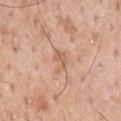notes: total-body-photography surveillance lesion; no biopsy
site: the chest
lesion diameter: ~3 mm (longest diameter)
patient: male, approximately 60 years of age
tile lighting: white-light
image-analysis metrics: a lesion color around L≈62 a*≈21 b*≈33 in CIELAB, a lesion–skin lightness drop of about 8, and a lesion-to-skin contrast of about 5.5 (normalized; higher = more distinct)
image source: 15 mm crop, total-body photography